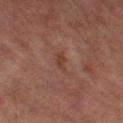<record>
  <biopsy_status>not biopsied; imaged during a skin examination</biopsy_status>
  <image>
    <source>total-body photography crop</source>
    <field_of_view_mm>15</field_of_view_mm>
  </image>
  <site>right thigh</site>
  <patient>
    <sex>female</sex>
    <age_approx>60</age_approx>
  </patient>
  <lighting>cross-polarized</lighting>
  <automated_metrics>
    <area_mm2_approx>3.0</area_mm2_approx>
    <eccentricity>0.9</eccentricity>
    <cielab_L>35</cielab_L>
    <cielab_a>20</cielab_a>
    <cielab_b>25</cielab_b>
    <vs_skin_darker_L>6.0</vs_skin_darker_L>
    <vs_skin_contrast_norm>7.0</vs_skin_contrast_norm>
    <border_irregularity_0_10>4.5</border_irregularity_0_10>
    <color_variation_0_10>0.0</color_variation_0_10>
    <nevus_likeness_0_100>0</nevus_likeness_0_100>
    <lesion_detection_confidence_0_100>100</lesion_detection_confidence_0_100>
  </automated_metrics>
</record>